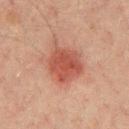follow-up=catalogued during a skin exam; not biopsied
image=15 mm crop, total-body photography
site=the chest
image-analysis metrics=an area of roughly 14 mm² and an eccentricity of roughly 0.6
lighting=cross-polarized illumination
patient=male, aged approximately 45